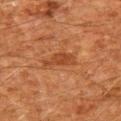biopsy status: catalogued during a skin exam; not biopsied
image: total-body-photography crop, ~15 mm field of view
subject: male, aged 58 to 62
location: the left upper arm